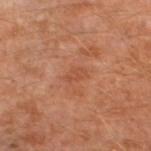| feature | finding |
|---|---|
| notes | catalogued during a skin exam; not biopsied |
| body site | the left lower leg |
| lesion size | ~2.5 mm (longest diameter) |
| subject | male, aged 28–32 |
| imaging modality | ~15 mm crop, total-body skin-cancer survey |